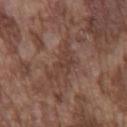| key | value |
|---|---|
| notes | total-body-photography surveillance lesion; no biopsy |
| subject | male, aged 73–77 |
| body site | the chest |
| acquisition | 15 mm crop, total-body photography |